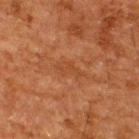follow-up — catalogued during a skin exam; not biopsied
size — about 3.5 mm
anatomic site — the back
image source — ~15 mm crop, total-body skin-cancer survey
patient — male, approximately 60 years of age
image-analysis metrics — a lesion color around L≈35 a*≈21 b*≈31 in CIELAB, a lesion–skin lightness drop of about 4, and a normalized border contrast of about 4.5
illumination — cross-polarized